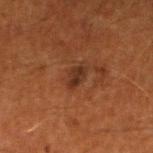The lesion was tiled from a total-body skin photograph and was not biopsied.
The lesion is located on the left lower leg.
The patient is a male in their 60s.
Cropped from a total-body skin-imaging series; the visible field is about 15 mm.